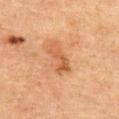• follow-up — imaged on a skin check; not biopsied
• illumination — cross-polarized illumination
• diameter — ≈4 mm
• site — the chest
• image — 15 mm crop, total-body photography
• subject — female, aged 58 to 62